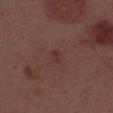<tbp_lesion>
<biopsy_status>not biopsied; imaged during a skin examination</biopsy_status>
<lesion_size>
  <long_diameter_mm_approx>1.5</long_diameter_mm_approx>
</lesion_size>
<lighting>white-light</lighting>
<site>upper back</site>
<patient>
  <sex>male</sex>
  <age_approx>55</age_approx>
</patient>
<image>
  <source>total-body photography crop</source>
  <field_of_view_mm>15</field_of_view_mm>
</image>
</tbp_lesion>